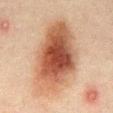Part of a total-body skin-imaging series; this lesion was reviewed on a skin check and was not flagged for biopsy. From the abdomen. Imaged with cross-polarized lighting. The subject is a male approximately 50 years of age. A region of skin cropped from a whole-body photographic capture, roughly 15 mm wide.